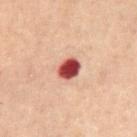<tbp_lesion>
<biopsy_status>not biopsied; imaged during a skin examination</biopsy_status>
<patient>
  <sex>male</sex>
  <age_approx>60</age_approx>
</patient>
<site>abdomen</site>
<lighting>cross-polarized</lighting>
<image>
  <source>total-body photography crop</source>
  <field_of_view_mm>15</field_of_view_mm>
</image>
</tbp_lesion>